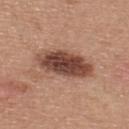The tile uses white-light illumination.
A region of skin cropped from a whole-body photographic capture, roughly 15 mm wide.
From the upper back.
A female patient in their mid-30s.
Measured at roughly 7 mm in maximum diameter.